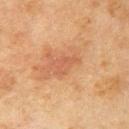imaging modality — total-body-photography crop, ~15 mm field of view
lesion diameter — ~3.5 mm (longest diameter)
subject — male, about 50 years old
body site — the arm
image-analysis metrics — a lesion area of about 5 mm², an outline eccentricity of about 0.8 (0 = round, 1 = elongated), and a shape-asymmetry score of about 0.45 (0 = symmetric); a normalized lesion–skin contrast near 4.5
lighting — cross-polarized